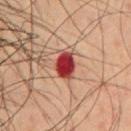Captured during whole-body skin photography for melanoma surveillance; the lesion was not biopsied. About 3.5 mm across. On the chest. Imaged with cross-polarized lighting. Cropped from a total-body skin-imaging series; the visible field is about 15 mm. A male subject, in their mid- to late 40s.